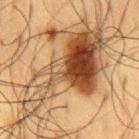Q: Is there a histopathology result?
A: no biopsy performed (imaged during a skin exam)
Q: Where on the body is the lesion?
A: the mid back
Q: Automated lesion metrics?
A: a border-irregularity index near 8.5/10 and internal color variation of about 10 on a 0–10 scale; a nevus-likeness score of about 100/100 and lesion-presence confidence of about 100/100
Q: How large is the lesion?
A: about 14.5 mm
Q: Illumination type?
A: cross-polarized illumination
Q: Patient demographics?
A: male, aged around 60
Q: How was this image acquired?
A: ~15 mm crop, total-body skin-cancer survey